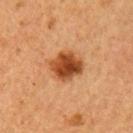The lesion was tiled from a total-body skin photograph and was not biopsied. The recorded lesion diameter is about 3.5 mm. The lesion is located on the left upper arm. The subject is a female aged approximately 60. A roughly 15 mm field-of-view crop from a total-body skin photograph.